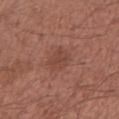No biopsy was performed on this lesion — it was imaged during a full skin examination and was not determined to be concerning.
A lesion tile, about 15 mm wide, cut from a 3D total-body photograph.
Automated tile analysis of the lesion measured an outline eccentricity of about 0.65 (0 = round, 1 = elongated) and a shape-asymmetry score of about 0.2 (0 = symmetric). And it measured a border-irregularity rating of about 2/10 and a color-variation rating of about 2/10.
The subject is a male about 40 years old.
On the right upper arm.
The tile uses white-light illumination.